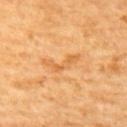The lesion was tiled from a total-body skin photograph and was not biopsied.
Located on the back.
A 15 mm crop from a total-body photograph taken for skin-cancer surveillance.
Longest diameter approximately 4 mm.
A male subject, aged 58 to 62.
The tile uses cross-polarized illumination.